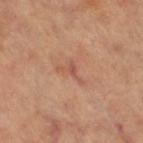The lesion was tiled from a total-body skin photograph and was not biopsied. The patient is a female aged approximately 60. The recorded lesion diameter is about 3.5 mm. The lesion-visualizer software estimated lesion-presence confidence of about 95/100. Located on the left leg. A region of skin cropped from a whole-body photographic capture, roughly 15 mm wide. This is a cross-polarized tile.